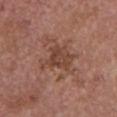Q: What is the imaging modality?
A: ~15 mm tile from a whole-body skin photo
Q: Who is the patient?
A: female, in their mid- to late 60s
Q: How was the tile lit?
A: white-light
Q: Where on the body is the lesion?
A: the chest